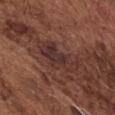<record>
  <biopsy_status>not biopsied; imaged during a skin examination</biopsy_status>
  <site>chest</site>
  <automated_metrics>
    <border_irregularity_0_10>6.0</border_irregularity_0_10>
    <color_variation_0_10>4.0</color_variation_0_10>
    <peripheral_color_asymmetry>1.0</peripheral_color_asymmetry>
  </automated_metrics>
  <image>
    <source>total-body photography crop</source>
    <field_of_view_mm>15</field_of_view_mm>
  </image>
  <lesion_size>
    <long_diameter_mm_approx>5.5</long_diameter_mm_approx>
  </lesion_size>
  <patient>
    <sex>male</sex>
    <age_approx>75</age_approx>
  </patient>
  <lighting>white-light</lighting>
</record>